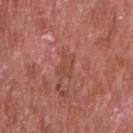No biopsy was performed on this lesion — it was imaged during a full skin examination and was not determined to be concerning.
Automated image analysis of the tile measured an automated nevus-likeness rating near 0 out of 100 and a lesion-detection confidence of about 70/100.
The tile uses white-light illumination.
A male subject, approximately 65 years of age.
A roughly 15 mm field-of-view crop from a total-body skin photograph.
Approximately 3 mm at its widest.
Located on the head or neck.